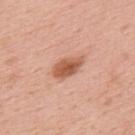lighting: white-light
image:
  source: total-body photography crop
  field_of_view_mm: 15
patient:
  sex: male
  age_approx: 60
site: upper back